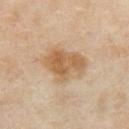Imaged during a routine full-body skin examination; the lesion was not biopsied and no histopathology is available.
The lesion is on the abdomen.
Captured under cross-polarized illumination.
A close-up tile cropped from a whole-body skin photograph, about 15 mm across.
A female patient aged 48–52.
The total-body-photography lesion software estimated an average lesion color of about L≈58 a*≈16 b*≈35 (CIELAB).
The lesion's longest dimension is about 5.5 mm.